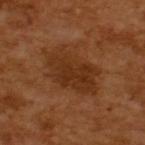Assessment:
Recorded during total-body skin imaging; not selected for excision or biopsy.